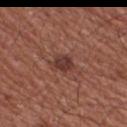Impression: Recorded during total-body skin imaging; not selected for excision or biopsy. Background: Cropped from a total-body skin-imaging series; the visible field is about 15 mm. Captured under white-light illumination. A male subject, in their mid- to late 60s. Measured at roughly 3 mm in maximum diameter. The lesion is located on the upper back.